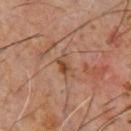Imaged during a routine full-body skin examination; the lesion was not biopsied and no histopathology is available. From the chest. This is a cross-polarized tile. A male patient, about 60 years old. Cropped from a whole-body photographic skin survey; the tile spans about 15 mm. Longest diameter approximately 3 mm. Automated image analysis of the tile measured a lesion area of about 4 mm² and a symmetry-axis asymmetry near 0.3. It also reported an average lesion color of about L≈44 a*≈19 b*≈30 (CIELAB), roughly 8 lightness units darker than nearby skin, and a normalized lesion–skin contrast near 7. And it measured border irregularity of about 3 on a 0–10 scale, a within-lesion color-variation index near 5/10, and radial color variation of about 1.5. It also reported a classifier nevus-likeness of about 0/100.